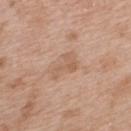Recorded during total-body skin imaging; not selected for excision or biopsy. A lesion tile, about 15 mm wide, cut from a 3D total-body photograph. The subject is a female in their 40s. The total-body-photography lesion software estimated a border-irregularity index near 5.5/10, a color-variation rating of about 2/10, and peripheral color asymmetry of about 0.5. The analysis additionally found a classifier nevus-likeness of about 0/100 and a lesion-detection confidence of about 100/100. From the upper back. The tile uses white-light illumination. About 3.5 mm across.